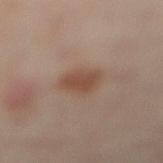Q: Is there a histopathology result?
A: imaged on a skin check; not biopsied
Q: How large is the lesion?
A: ≈3.5 mm
Q: What lighting was used for the tile?
A: cross-polarized illumination
Q: Lesion location?
A: the left leg
Q: Patient demographics?
A: female, aged 38–42
Q: How was this image acquired?
A: 15 mm crop, total-body photography